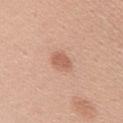Part of a total-body skin-imaging series; this lesion was reviewed on a skin check and was not flagged for biopsy.
From the back.
A lesion tile, about 15 mm wide, cut from a 3D total-body photograph.
The tile uses white-light illumination.
Measured at roughly 3 mm in maximum diameter.
A female subject, aged 38–42.